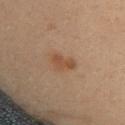<case>
  <biopsy_status>not biopsied; imaged during a skin examination</biopsy_status>
  <site>back</site>
  <lighting>cross-polarized</lighting>
  <lesion_size>
    <long_diameter_mm_approx>3.0</long_diameter_mm_approx>
  </lesion_size>
  <patient>
    <sex>female</sex>
    <age_approx>35</age_approx>
  </patient>
  <image>
    <source>total-body photography crop</source>
    <field_of_view_mm>15</field_of_view_mm>
  </image>
</case>